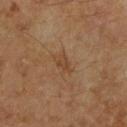Impression:
No biopsy was performed on this lesion — it was imaged during a full skin examination and was not determined to be concerning.